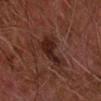Impression: Recorded during total-body skin imaging; not selected for excision or biopsy. Acquisition and patient details: Automated image analysis of the tile measured a lesion area of about 8.5 mm², an eccentricity of roughly 0.75, and two-axis asymmetry of about 0.55. The analysis additionally found a border-irregularity rating of about 6/10 and a peripheral color-asymmetry measure near 0.5. On the left forearm. The lesion's longest dimension is about 4.5 mm. A male patient, in their mid-70s. Cropped from a total-body skin-imaging series; the visible field is about 15 mm.